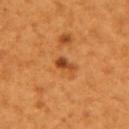A female patient in their mid- to late 50s. Longest diameter approximately 2.5 mm. Automated image analysis of the tile measured an average lesion color of about L≈48 a*≈29 b*≈44 (CIELAB) and roughly 12 lightness units darker than nearby skin. The software also gave a border-irregularity index near 3.5/10, internal color variation of about 2 on a 0–10 scale, and peripheral color asymmetry of about 0.5. Imaged with cross-polarized lighting. Located on the left upper arm. A close-up tile cropped from a whole-body skin photograph, about 15 mm across.